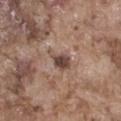A roughly 15 mm field-of-view crop from a total-body skin photograph. The lesion-visualizer software estimated a lesion area of about 5 mm² and an eccentricity of roughly 0.8. A male subject about 75 years old. The lesion is located on the abdomen. The recorded lesion diameter is about 3.5 mm.